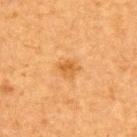The lesion was photographed on a routine skin check and not biopsied; there is no pathology result. On the back. The lesion-visualizer software estimated an area of roughly 4.5 mm² and a symmetry-axis asymmetry near 0.25. It also reported internal color variation of about 2.5 on a 0–10 scale. This image is a 15 mm lesion crop taken from a total-body photograph. The subject is a female about 40 years old.